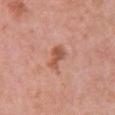Assessment:
Imaged during a routine full-body skin examination; the lesion was not biopsied and no histopathology is available.
Background:
A female subject, in their 40s. The lesion-visualizer software estimated a nevus-likeness score of about 15/100 and lesion-presence confidence of about 100/100. From the chest. The lesion's longest dimension is about 3.5 mm. This is a white-light tile. A 15 mm close-up tile from a total-body photography series done for melanoma screening.